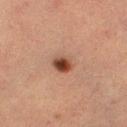biopsy status: catalogued during a skin exam; not biopsied | tile lighting: cross-polarized illumination | size: about 3.5 mm | anatomic site: the left thigh | image-analysis metrics: a mean CIELAB color near L≈40 a*≈18 b*≈25, roughly 9 lightness units darker than nearby skin, and a lesion-to-skin contrast of about 8 (normalized; higher = more distinct) | patient: female, aged 53 to 57 | acquisition: ~15 mm crop, total-body skin-cancer survey.